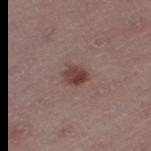Imaged during a routine full-body skin examination; the lesion was not biopsied and no histopathology is available.
The lesion is on the left thigh.
Captured under white-light illumination.
Longest diameter approximately 3 mm.
A 15 mm crop from a total-body photograph taken for skin-cancer surveillance.
A female subject, about 45 years old.
Automated tile analysis of the lesion measured an area of roughly 5 mm², a shape eccentricity near 0.55, and a shape-asymmetry score of about 0.25 (0 = symmetric). The analysis additionally found a mean CIELAB color near L≈41 a*≈19 b*≈21, about 11 CIELAB-L* units darker than the surrounding skin, and a lesion-to-skin contrast of about 9 (normalized; higher = more distinct).